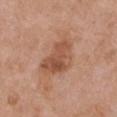Recorded during total-body skin imaging; not selected for excision or biopsy. A 15 mm close-up tile from a total-body photography series done for melanoma screening. The subject is a female in their 40s. The lesion is on the chest. Imaged with white-light lighting.